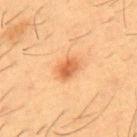– tile lighting · cross-polarized
– automated metrics · an area of roughly 5 mm², an eccentricity of roughly 0.7, and two-axis asymmetry of about 0.15; a classifier nevus-likeness of about 95/100 and a lesion-detection confidence of about 100/100
– location · the chest
– diameter · ~3 mm (longest diameter)
– image source · ~15 mm crop, total-body skin-cancer survey
– patient · male, about 55 years old